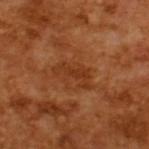Captured during whole-body skin photography for melanoma surveillance; the lesion was not biopsied. A male subject, approximately 65 years of age. Approximately 4.5 mm at its widest. The tile uses cross-polarized illumination. An algorithmic analysis of the crop reported a footprint of about 5.5 mm², a shape eccentricity near 0.9, and a symmetry-axis asymmetry near 0.55. And it measured an average lesion color of about L≈35 a*≈26 b*≈36 (CIELAB), a lesion–skin lightness drop of about 7, and a normalized lesion–skin contrast near 6.5. The analysis additionally found border irregularity of about 7.5 on a 0–10 scale, internal color variation of about 0.5 on a 0–10 scale, and a peripheral color-asymmetry measure near 0. A 15 mm close-up tile from a total-body photography series done for melanoma screening.